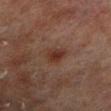Captured during whole-body skin photography for melanoma surveillance; the lesion was not biopsied.
Located on the left lower leg.
The total-body-photography lesion software estimated a border-irregularity rating of about 2/10, a color-variation rating of about 3.5/10, and a peripheral color-asymmetry measure near 1. It also reported a lesion-detection confidence of about 100/100.
A male subject, approximately 70 years of age.
A 15 mm close-up extracted from a 3D total-body photography capture.
Measured at roughly 2.5 mm in maximum diameter.